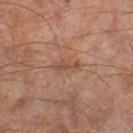On the right lower leg. A lesion tile, about 15 mm wide, cut from a 3D total-body photograph. A male subject about 65 years old. Measured at roughly 3 mm in maximum diameter. The tile uses cross-polarized illumination.